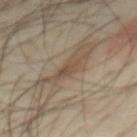| key | value |
|---|---|
| follow-up | imaged on a skin check; not biopsied |
| site | the mid back |
| subject | male, aged 38–42 |
| imaging modality | total-body-photography crop, ~15 mm field of view |
| image-analysis metrics | border irregularity of about 3 on a 0–10 scale, internal color variation of about 2.5 on a 0–10 scale, and peripheral color asymmetry of about 0.5; a detector confidence of about 70 out of 100 that the crop contains a lesion |
| lesion diameter | ~3.5 mm (longest diameter) |